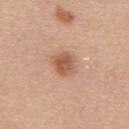acquisition: total-body-photography crop, ~15 mm field of view; site: the mid back; lighting: white-light; patient: female, aged approximately 65; lesion size: ≈3 mm.